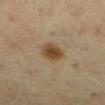* biopsy status — total-body-photography surveillance lesion; no biopsy
* image source — ~15 mm tile from a whole-body skin photo
* illumination — cross-polarized illumination
* subject — female, aged 53 to 57
* location — the left lower leg
* size — ~3 mm (longest diameter)